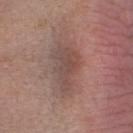Clinical impression: Recorded during total-body skin imaging; not selected for excision or biopsy. Background: Located on the head or neck. The tile uses white-light illumination. A 15 mm crop from a total-body photograph taken for skin-cancer surveillance. A female patient, approximately 40 years of age. The lesion-visualizer software estimated roughly 8 lightness units darker than nearby skin and a lesion-to-skin contrast of about 6 (normalized; higher = more distinct). And it measured a border-irregularity rating of about 4.5/10, a color-variation rating of about 3.5/10, and a peripheral color-asymmetry measure near 1.